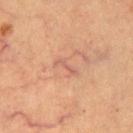No biopsy was performed on this lesion — it was imaged during a full skin examination and was not determined to be concerning. The tile uses cross-polarized illumination. A male patient, about 65 years old. A close-up tile cropped from a whole-body skin photograph, about 15 mm across. Automated tile analysis of the lesion measured a lesion color around L≈55 a*≈23 b*≈28 in CIELAB, a lesion–skin lightness drop of about 7, and a lesion-to-skin contrast of about 5.5 (normalized; higher = more distinct). And it measured an automated nevus-likeness rating near 0 out of 100 and a lesion-detection confidence of about 95/100. The lesion is on the mid back.